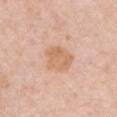No biopsy was performed on this lesion — it was imaged during a full skin examination and was not determined to be concerning.
A 15 mm close-up extracted from a 3D total-body photography capture.
The total-body-photography lesion software estimated roughly 8 lightness units darker than nearby skin and a normalized lesion–skin contrast near 6.5.
This is a white-light tile.
The lesion is located on the front of the torso.
A female subject, roughly 50 years of age.